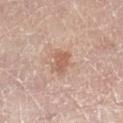Q: Was this lesion biopsied?
A: total-body-photography surveillance lesion; no biopsy
Q: What are the patient's age and sex?
A: male, aged 78 to 82
Q: Illumination type?
A: white-light illumination
Q: What is the anatomic site?
A: the leg
Q: Automated lesion metrics?
A: a lesion area of about 5 mm² and a shape-asymmetry score of about 0.25 (0 = symmetric); a border-irregularity rating of about 2.5/10, internal color variation of about 2.5 on a 0–10 scale, and peripheral color asymmetry of about 1
Q: What kind of image is this?
A: ~15 mm crop, total-body skin-cancer survey
Q: Lesion size?
A: about 2.5 mm